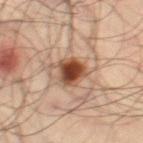Assessment: The lesion was photographed on a routine skin check and not biopsied; there is no pathology result. Context: From the leg. Captured under cross-polarized illumination. A close-up tile cropped from a whole-body skin photograph, about 15 mm across. A male subject, about 35 years old.